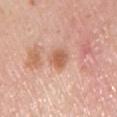Assessment: The lesion was photographed on a routine skin check and not biopsied; there is no pathology result. Image and clinical context: On the right upper arm. The patient is a male aged around 60. An algorithmic analysis of the crop reported a lesion area of about 6 mm², a shape eccentricity near 0.55, and a symmetry-axis asymmetry near 0.25. And it measured a mean CIELAB color near L≈61 a*≈24 b*≈32 and a normalized lesion–skin contrast near 7.5. It also reported a border-irregularity index near 2/10 and internal color variation of about 3 on a 0–10 scale. The analysis additionally found a lesion-detection confidence of about 100/100. About 3 mm across. A 15 mm close-up extracted from a 3D total-body photography capture. The tile uses white-light illumination.